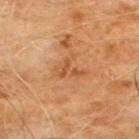This lesion was catalogued during total-body skin photography and was not selected for biopsy.
An algorithmic analysis of the crop reported a lesion area of about 6 mm² and a shape-asymmetry score of about 0.65 (0 = symmetric). And it measured a mean CIELAB color near L≈52 a*≈24 b*≈39, a lesion–skin lightness drop of about 7, and a lesion-to-skin contrast of about 5.5 (normalized; higher = more distinct). It also reported a border-irregularity index near 7.5/10, a within-lesion color-variation index near 3.5/10, and radial color variation of about 1. And it measured an automated nevus-likeness rating near 0 out of 100 and a lesion-detection confidence of about 100/100.
The lesion is on the chest.
The lesion's longest dimension is about 3.5 mm.
Cropped from a total-body skin-imaging series; the visible field is about 15 mm.
The patient is a male aged around 60.